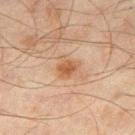Assessment: Captured during whole-body skin photography for melanoma surveillance; the lesion was not biopsied. Clinical summary: From the leg. A male patient aged 43 to 47. The total-body-photography lesion software estimated an area of roughly 5 mm², an eccentricity of roughly 0.35, and two-axis asymmetry of about 0.35. This is a cross-polarized tile. A 15 mm close-up extracted from a 3D total-body photography capture. The lesion's longest dimension is about 2.5 mm.